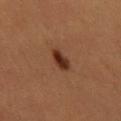| feature | finding |
|---|---|
| biopsy status | catalogued during a skin exam; not biopsied |
| acquisition | total-body-photography crop, ~15 mm field of view |
| subject | female, aged approximately 45 |
| site | the leg |
| size | about 3 mm |